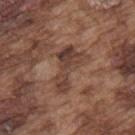Clinical summary: Imaged with white-light lighting. The lesion is on the upper back. Automated tile analysis of the lesion measured an average lesion color of about L≈40 a*≈19 b*≈25 (CIELAB), about 8 CIELAB-L* units darker than the surrounding skin, and a normalized lesion–skin contrast near 7.5. And it measured a border-irregularity index near 6/10 and a peripheral color-asymmetry measure near 1.5. It also reported a nevus-likeness score of about 0/100 and a lesion-detection confidence of about 95/100. Longest diameter approximately 5.5 mm. A male patient in their mid- to late 70s. A 15 mm crop from a total-body photograph taken for skin-cancer surveillance.